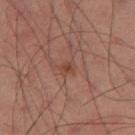* follow-up — no biopsy performed (imaged during a skin exam)